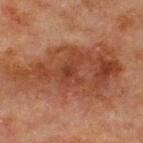biopsy status = no biopsy performed (imaged during a skin exam); automated lesion analysis = a border-irregularity rating of about 6.5/10 and a peripheral color-asymmetry measure near 1.5; subject = male, roughly 65 years of age; location = the chest; image = ~15 mm crop, total-body skin-cancer survey; illumination = cross-polarized.